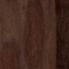Notes:
- site · the abdomen
- image source · ~15 mm crop, total-body skin-cancer survey
- lighting · white-light
- diameter · ≈11 mm
- patient · male, aged 68–72